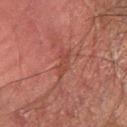Case summary:
- body site: the right forearm
- subject: male, aged 68–72
- automated metrics: a footprint of about 4 mm² and a shape-asymmetry score of about 0.35 (0 = symmetric); a border-irregularity rating of about 4/10, a color-variation rating of about 1.5/10, and radial color variation of about 0.5
- tile lighting: cross-polarized
- image source: total-body-photography crop, ~15 mm field of view
- lesion diameter: ≈3 mm